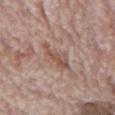Notes:
• notes — catalogued during a skin exam; not biopsied
• imaging modality — 15 mm crop, total-body photography
• diameter — about 4 mm
• subject — male, aged 68–72
• lighting — white-light
• anatomic site — the back